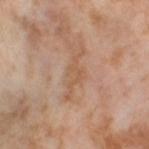Part of a total-body skin-imaging series; this lesion was reviewed on a skin check and was not flagged for biopsy.
Imaged with cross-polarized lighting.
Automated tile analysis of the lesion measured an area of roughly 5 mm² and a shape-asymmetry score of about 0.45 (0 = symmetric). The software also gave border irregularity of about 8.5 on a 0–10 scale, a within-lesion color-variation index near 0.5/10, and radial color variation of about 0.
A region of skin cropped from a whole-body photographic capture, roughly 15 mm wide.
A female patient approximately 55 years of age.
On the left thigh.